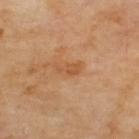Captured during whole-body skin photography for melanoma surveillance; the lesion was not biopsied. Cropped from a total-body skin-imaging series; the visible field is about 15 mm. Automated image analysis of the tile measured an average lesion color of about L≈55 a*≈24 b*≈39 (CIELAB), about 7 CIELAB-L* units darker than the surrounding skin, and a lesion-to-skin contrast of about 6 (normalized; higher = more distinct). And it measured a border-irregularity index near 4/10. And it measured a nevus-likeness score of about 0/100 and a lesion-detection confidence of about 100/100. The tile uses cross-polarized illumination. Approximately 3 mm at its widest. The lesion is on the upper back.